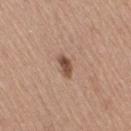| key | value |
|---|---|
| follow-up | no biopsy performed (imaged during a skin exam) |
| subject | female, in their mid- to late 50s |
| illumination | white-light illumination |
| size | ~3 mm (longest diameter) |
| image | ~15 mm tile from a whole-body skin photo |
| anatomic site | the right thigh |